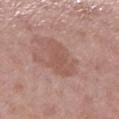Case summary:
* follow-up · total-body-photography surveillance lesion; no biopsy
* illumination · white-light illumination
* subject · female, about 50 years old
* location · the right lower leg
* image-analysis metrics · a lesion color around L≈54 a*≈21 b*≈25 in CIELAB, roughly 7 lightness units darker than nearby skin, and a normalized border contrast of about 5.5
* image · ~15 mm tile from a whole-body skin photo